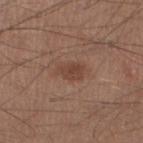Q: Was this lesion biopsied?
A: no biopsy performed (imaged during a skin exam)
Q: What is the imaging modality?
A: total-body-photography crop, ~15 mm field of view
Q: Lesion location?
A: the left lower leg
Q: Patient demographics?
A: male, roughly 30 years of age
Q: What lighting was used for the tile?
A: white-light